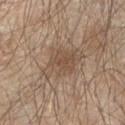{"biopsy_status": "not biopsied; imaged during a skin examination", "patient": {"sex": "male", "age_approx": 65}, "lighting": "white-light", "lesion_size": {"long_diameter_mm_approx": 5.5}, "site": "left upper arm", "automated_metrics": {"area_mm2_approx": 13.0, "eccentricity": 0.75, "lesion_detection_confidence_0_100": 100}, "image": {"source": "total-body photography crop", "field_of_view_mm": 15}}